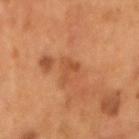{
  "biopsy_status": "not biopsied; imaged during a skin examination"
}